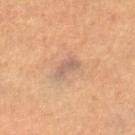| feature | finding |
|---|---|
| automated metrics | a color-variation rating of about 1.5/10 and peripheral color asymmetry of about 0.5; an automated nevus-likeness rating near 0 out of 100 |
| subject | female, aged 58–62 |
| illumination | cross-polarized illumination |
| body site | the right thigh |
| acquisition | ~15 mm tile from a whole-body skin photo |